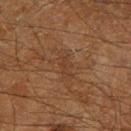Case summary:
– workup · catalogued during a skin exam; not biopsied
– automated metrics · an area of roughly 4.5 mm², a shape eccentricity near 0.9, and two-axis asymmetry of about 0.3; a mean CIELAB color near L≈29 a*≈15 b*≈24, roughly 4 lightness units darker than nearby skin, and a lesion-to-skin contrast of about 4.5 (normalized; higher = more distinct); a border-irregularity index near 4/10, a within-lesion color-variation index near 1/10, and peripheral color asymmetry of about 0
– acquisition · ~15 mm crop, total-body skin-cancer survey
– patient · male, aged 58–62
– site · the right lower leg
– illumination · cross-polarized illumination